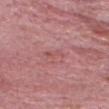Recorded during total-body skin imaging; not selected for excision or biopsy.
A male subject, about 65 years old.
On the head or neck.
Cropped from a total-body skin-imaging series; the visible field is about 15 mm.
This is a white-light tile.
Longest diameter approximately 2.5 mm.
Automated image analysis of the tile measured a lesion color around L≈53 a*≈28 b*≈22 in CIELAB, a lesion–skin lightness drop of about 6, and a normalized lesion–skin contrast near 4.5. And it measured a border-irregularity index near 4/10, internal color variation of about 0 on a 0–10 scale, and radial color variation of about 0. It also reported an automated nevus-likeness rating near 0 out of 100 and a detector confidence of about 95 out of 100 that the crop contains a lesion.